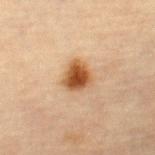Recorded during total-body skin imaging; not selected for excision or biopsy. Measured at roughly 3 mm in maximum diameter. The lesion is located on the right thigh. A 15 mm close-up extracted from a 3D total-body photography capture. The total-body-photography lesion software estimated a footprint of about 7.5 mm². It also reported roughly 15 lightness units darker than nearby skin. The software also gave lesion-presence confidence of about 100/100. The patient is a female aged 63–67.